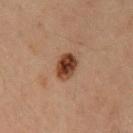Captured during whole-body skin photography for melanoma surveillance; the lesion was not biopsied. Located on the chest. Cropped from a total-body skin-imaging series; the visible field is about 15 mm. A male subject, about 60 years old. The tile uses cross-polarized illumination. About 3.5 mm across.